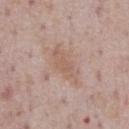Part of a total-body skin-imaging series; this lesion was reviewed on a skin check and was not flagged for biopsy. Imaged with white-light lighting. On the chest. About 5 mm across. A male patient, aged approximately 75. A roughly 15 mm field-of-view crop from a total-body skin photograph. An algorithmic analysis of the crop reported a lesion color around L≈59 a*≈18 b*≈26 in CIELAB, about 7 CIELAB-L* units darker than the surrounding skin, and a normalized border contrast of about 5.5.